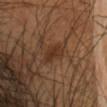site=the head or neck
lighting=cross-polarized illumination
subject=male, in their 40s
lesion size=≈3 mm
automated lesion analysis=a lesion color around L≈33 a*≈18 b*≈29 in CIELAB and roughly 7 lightness units darker than nearby skin; internal color variation of about 2.5 on a 0–10 scale and peripheral color asymmetry of about 1
acquisition=15 mm crop, total-body photography